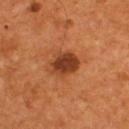Q: How was this image acquired?
A: 15 mm crop, total-body photography
Q: Automated lesion metrics?
A: a mean CIELAB color near L≈37 a*≈26 b*≈34, roughly 12 lightness units darker than nearby skin, and a normalized lesion–skin contrast near 10; border irregularity of about 1.5 on a 0–10 scale and peripheral color asymmetry of about 1; a classifier nevus-likeness of about 80/100
Q: What lighting was used for the tile?
A: cross-polarized illumination
Q: How large is the lesion?
A: ~3.5 mm (longest diameter)
Q: Who is the patient?
A: male, in their mid- to late 50s
Q: What is the anatomic site?
A: the upper back